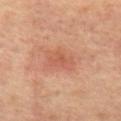Impression:
No biopsy was performed on this lesion — it was imaged during a full skin examination and was not determined to be concerning.
Context:
This is a cross-polarized tile. A female subject, aged approximately 45. Measured at roughly 3.5 mm in maximum diameter. The lesion is located on the mid back. A region of skin cropped from a whole-body photographic capture, roughly 15 mm wide.